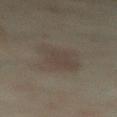Q: Was a biopsy performed?
A: no biopsy performed (imaged during a skin exam)
Q: What is the lesion's diameter?
A: ~5.5 mm (longest diameter)
Q: How was this image acquired?
A: ~15 mm tile from a whole-body skin photo
Q: Lesion location?
A: the abdomen
Q: Patient demographics?
A: female, roughly 35 years of age